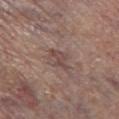notes=catalogued during a skin exam; not biopsied
lesion size=~3 mm (longest diameter)
site=the leg
image source=~15 mm tile from a whole-body skin photo
illumination=white-light illumination
patient=male, approximately 70 years of age